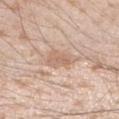{
  "biopsy_status": "not biopsied; imaged during a skin examination",
  "lesion_size": {
    "long_diameter_mm_approx": 4.0
  },
  "image": {
    "source": "total-body photography crop",
    "field_of_view_mm": 15
  },
  "automated_metrics": {
    "area_mm2_approx": 9.0,
    "shape_asymmetry": 0.25,
    "cielab_L": 65,
    "cielab_a": 17,
    "cielab_b": 29,
    "vs_skin_darker_L": 7.0,
    "vs_skin_contrast_norm": 5.0,
    "border_irregularity_0_10": 3.0,
    "peripheral_color_asymmetry": 1.0
  },
  "lighting": "white-light",
  "patient": {
    "sex": "male",
    "age_approx": 25
  },
  "site": "arm"
}